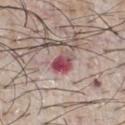Assessment:
This lesion was catalogued during total-body skin photography and was not selected for biopsy.
Context:
A 15 mm crop from a total-body photograph taken for skin-cancer surveillance. This is a white-light tile. A male subject, roughly 65 years of age. The total-body-photography lesion software estimated a footprint of about 5.5 mm², a shape eccentricity near 0.7, and a symmetry-axis asymmetry near 0.25. The software also gave a lesion color around L≈49 a*≈28 b*≈18 in CIELAB, about 15 CIELAB-L* units darker than the surrounding skin, and a normalized border contrast of about 10.5. The lesion is on the front of the torso. Measured at roughly 3 mm in maximum diameter.